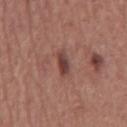biopsy_status: not biopsied; imaged during a skin examination